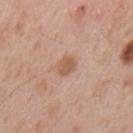| field | value |
|---|---|
| follow-up | imaged on a skin check; not biopsied |
| illumination | white-light illumination |
| anatomic site | the mid back |
| lesion size | ~2.5 mm (longest diameter) |
| automated metrics | a border-irregularity index near 1.5/10, a color-variation rating of about 2/10, and a peripheral color-asymmetry measure near 0.5 |
| acquisition | ~15 mm tile from a whole-body skin photo |
| patient | male, aged around 55 |